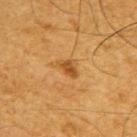<record>
<biopsy_status>not biopsied; imaged during a skin examination</biopsy_status>
<site>back</site>
<patient>
  <sex>male</sex>
  <age_approx>65</age_approx>
</patient>
<lighting>cross-polarized</lighting>
<image>
  <source>total-body photography crop</source>
  <field_of_view_mm>15</field_of_view_mm>
</image>
</record>